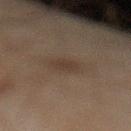Q: Was this lesion biopsied?
A: imaged on a skin check; not biopsied
Q: What lighting was used for the tile?
A: cross-polarized illumination
Q: Automated lesion metrics?
A: a shape eccentricity near 0.8; a detector confidence of about 100 out of 100 that the crop contains a lesion
Q: Who is the patient?
A: male, aged 43 to 47
Q: How was this image acquired?
A: 15 mm crop, total-body photography
Q: What is the lesion's diameter?
A: about 2.5 mm
Q: What is the anatomic site?
A: the left lower leg